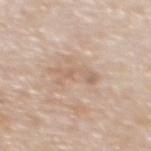No biopsy was performed on this lesion — it was imaged during a full skin examination and was not determined to be concerning. A female subject aged 48–52. An algorithmic analysis of the crop reported an area of roughly 5.5 mm², an outline eccentricity of about 0.95 (0 = round, 1 = elongated), and a symmetry-axis asymmetry near 0.5. And it measured a mean CIELAB color near L≈63 a*≈16 b*≈29, roughly 7 lightness units darker than nearby skin, and a normalized border contrast of about 5. Located on the upper back. This is a white-light tile. A 15 mm crop from a total-body photograph taken for skin-cancer surveillance.